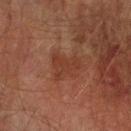biopsy_status: not biopsied; imaged during a skin examination
patient:
  sex: male
  age_approx: 75
image:
  source: total-body photography crop
  field_of_view_mm: 15
site: right forearm